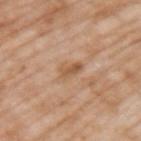Impression: Imaged during a routine full-body skin examination; the lesion was not biopsied and no histopathology is available. Context: This is a white-light tile. The subject is a male aged 58–62. Automated image analysis of the tile measured an average lesion color of about L≈56 a*≈20 b*≈34 (CIELAB), about 9 CIELAB-L* units darker than the surrounding skin, and a normalized border contrast of about 6.5. And it measured internal color variation of about 2.5 on a 0–10 scale and peripheral color asymmetry of about 1. The analysis additionally found an automated nevus-likeness rating near 0 out of 100 and a lesion-detection confidence of about 100/100. Located on the upper back. Approximately 3 mm at its widest. A 15 mm close-up extracted from a 3D total-body photography capture.